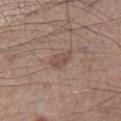Located on the leg.
A male patient, in their mid-50s.
A 15 mm crop from a total-body photograph taken for skin-cancer surveillance.
This is a white-light tile.
The lesion's longest dimension is about 3 mm.